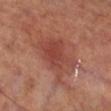Clinical impression:
This lesion was catalogued during total-body skin photography and was not selected for biopsy.
Acquisition and patient details:
A 15 mm crop from a total-body photograph taken for skin-cancer surveillance. Automated image analysis of the tile measured a lesion–skin lightness drop of about 8 and a lesion-to-skin contrast of about 6.5 (normalized; higher = more distinct). The patient is a male aged 68–72. Approximately 5.5 mm at its widest. The tile uses cross-polarized illumination. Located on the right lower leg.